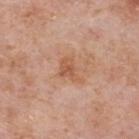<record>
  <biopsy_status>not biopsied; imaged during a skin examination</biopsy_status>
  <site>back</site>
  <image>
    <source>total-body photography crop</source>
    <field_of_view_mm>15</field_of_view_mm>
  </image>
  <patient>
    <sex>male</sex>
    <age_approx>75</age_approx>
  </patient>
</record>